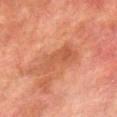Clinical impression:
The lesion was photographed on a routine skin check and not biopsied; there is no pathology result.
Background:
A region of skin cropped from a whole-body photographic capture, roughly 15 mm wide. This is a cross-polarized tile. A male patient, aged around 75. The lesion's longest dimension is about 5.5 mm. Located on the right lower leg.